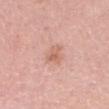Q: What did automated image analysis measure?
A: a footprint of about 4 mm², a shape eccentricity near 0.6, and a shape-asymmetry score of about 0.3 (0 = symmetric)
Q: What lighting was used for the tile?
A: white-light illumination
Q: Patient demographics?
A: female, about 30 years old
Q: Lesion location?
A: the head or neck
Q: What is the imaging modality?
A: 15 mm crop, total-body photography